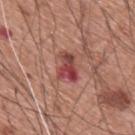<lesion>
  <image>
    <source>total-body photography crop</source>
    <field_of_view_mm>15</field_of_view_mm>
  </image>
  <site>upper back</site>
  <patient>
    <sex>male</sex>
    <age_approx>60</age_approx>
  </patient>
</lesion>